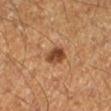Assessment:
Imaged during a routine full-body skin examination; the lesion was not biopsied and no histopathology is available.
Clinical summary:
On the right lower leg. This is a cross-polarized tile. Measured at roughly 3 mm in maximum diameter. Cropped from a whole-body photographic skin survey; the tile spans about 15 mm. The subject is a male aged approximately 60.